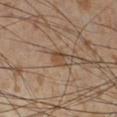- biopsy status · total-body-photography surveillance lesion; no biopsy
- imaging modality · 15 mm crop, total-body photography
- illumination · cross-polarized
- size · ≈2.5 mm
- anatomic site · the right lower leg
- automated metrics · an area of roughly 4 mm², an outline eccentricity of about 0.6 (0 = round, 1 = elongated), and a symmetry-axis asymmetry near 0.2; an average lesion color of about L≈47 a*≈17 b*≈30 (CIELAB), about 8 CIELAB-L* units darker than the surrounding skin, and a normalized lesion–skin contrast near 6.5; border irregularity of about 2 on a 0–10 scale and peripheral color asymmetry of about 1.5; an automated nevus-likeness rating near 5 out of 100 and a detector confidence of about 100 out of 100 that the crop contains a lesion
- patient · male, roughly 55 years of age